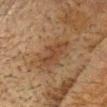{
  "biopsy_status": "not biopsied; imaged during a skin examination",
  "patient": {
    "sex": "male",
    "age_approx": 75
  },
  "image": {
    "source": "total-body photography crop",
    "field_of_view_mm": 15
  },
  "site": "head or neck"
}